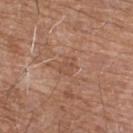| feature | finding |
|---|---|
| follow-up | total-body-photography surveillance lesion; no biopsy |
| location | the chest |
| patient | male, aged 58 to 62 |
| image | 15 mm crop, total-body photography |